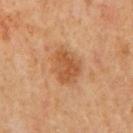Q: Was this lesion biopsied?
A: total-body-photography surveillance lesion; no biopsy
Q: What is the lesion's diameter?
A: about 4.5 mm
Q: How was this image acquired?
A: ~15 mm tile from a whole-body skin photo
Q: Who is the patient?
A: male, about 65 years old
Q: What is the anatomic site?
A: the mid back
Q: What did automated image analysis measure?
A: an area of roughly 11 mm², an eccentricity of roughly 0.65, and a symmetry-axis asymmetry near 0.2; a border-irregularity rating of about 2/10 and peripheral color asymmetry of about 1; a classifier nevus-likeness of about 30/100